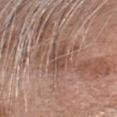Case summary:
- follow-up: total-body-photography surveillance lesion; no biopsy
- body site: the head or neck
- automated lesion analysis: a lesion area of about 5.5 mm², an eccentricity of roughly 0.75, and a shape-asymmetry score of about 0.3 (0 = symmetric); a border-irregularity index near 4/10
- lighting: white-light illumination
- subject: male, aged 73–77
- image: 15 mm crop, total-body photography